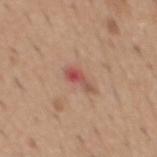The lesion was photographed on a routine skin check and not biopsied; there is no pathology result. The lesion is located on the mid back. Imaged with white-light lighting. A male subject, aged approximately 40. Measured at roughly 3 mm in maximum diameter. A lesion tile, about 15 mm wide, cut from a 3D total-body photograph.